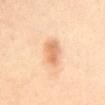Q: How large is the lesion?
A: ~5 mm (longest diameter)
Q: Illumination type?
A: cross-polarized illumination
Q: How was this image acquired?
A: ~15 mm tile from a whole-body skin photo
Q: Lesion location?
A: the mid back
Q: Patient demographics?
A: female, roughly 40 years of age